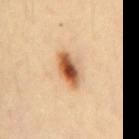The lesion was photographed on a routine skin check and not biopsied; there is no pathology result. A female subject aged 33–37. Located on the mid back. Imaged with cross-polarized lighting. A region of skin cropped from a whole-body photographic capture, roughly 15 mm wide.